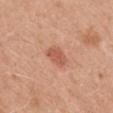Impression: Captured during whole-body skin photography for melanoma surveillance; the lesion was not biopsied. Acquisition and patient details: The subject is a male aged approximately 30. A lesion tile, about 15 mm wide, cut from a 3D total-body photograph. The total-body-photography lesion software estimated a lesion color around L≈57 a*≈26 b*≈32 in CIELAB. It also reported a border-irregularity rating of about 2/10, internal color variation of about 2 on a 0–10 scale, and a peripheral color-asymmetry measure near 1. The tile uses white-light illumination. On the mid back.